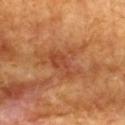The lesion was tiled from a total-body skin photograph and was not biopsied. The lesion-visualizer software estimated a footprint of about 15 mm² and a shape eccentricity near 0.7. And it measured an average lesion color of about L≈50 a*≈27 b*≈38 (CIELAB), roughly 8 lightness units darker than nearby skin, and a normalized lesion–skin contrast near 6. The software also gave a detector confidence of about 100 out of 100 that the crop contains a lesion. The subject is a female roughly 75 years of age. The recorded lesion diameter is about 6 mm. On the chest. The tile uses cross-polarized illumination. A region of skin cropped from a whole-body photographic capture, roughly 15 mm wide.